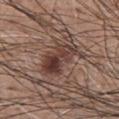Assessment: Captured during whole-body skin photography for melanoma surveillance; the lesion was not biopsied. Acquisition and patient details: A roughly 15 mm field-of-view crop from a total-body skin photograph. The tile uses white-light illumination. Located on the chest. Automated tile analysis of the lesion measured an average lesion color of about L≈39 a*≈18 b*≈22 (CIELAB), about 11 CIELAB-L* units darker than the surrounding skin, and a normalized lesion–skin contrast near 9.5. And it measured a border-irregularity rating of about 4.5/10, a within-lesion color-variation index near 8/10, and radial color variation of about 3.5. The software also gave a classifier nevus-likeness of about 90/100 and a lesion-detection confidence of about 100/100. The lesion's longest dimension is about 5.5 mm. The subject is a male about 60 years old.